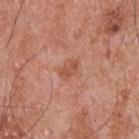Clinical impression:
Part of a total-body skin-imaging series; this lesion was reviewed on a skin check and was not flagged for biopsy.
Context:
Imaged with white-light lighting. The lesion is located on the upper back. A male patient, aged around 55. A 15 mm crop from a total-body photograph taken for skin-cancer surveillance. An algorithmic analysis of the crop reported a shape eccentricity near 0.8 and two-axis asymmetry of about 0.25. The software also gave a normalized border contrast of about 6.5. The analysis additionally found a classifier nevus-likeness of about 0/100 and lesion-presence confidence of about 100/100. Approximately 2.5 mm at its widest.